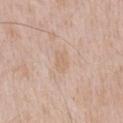follow-up — total-body-photography surveillance lesion; no biopsy | illumination — white-light | location — the chest | patient — male, aged around 50 | acquisition — total-body-photography crop, ~15 mm field of view.